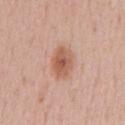Assessment: This lesion was catalogued during total-body skin photography and was not selected for biopsy. Clinical summary: Longest diameter approximately 4.5 mm. The subject is a male in their mid- to late 50s. A region of skin cropped from a whole-body photographic capture, roughly 15 mm wide. This is a white-light tile. The lesion is located on the chest. Automated image analysis of the tile measured a lesion area of about 9 mm², an eccentricity of roughly 0.85, and a shape-asymmetry score of about 0.1 (0 = symmetric). It also reported a border-irregularity rating of about 1.5/10, a within-lesion color-variation index near 5/10, and a peripheral color-asymmetry measure near 1.5.